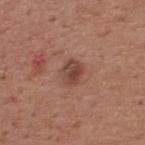notes: no biopsy performed (imaged during a skin exam) | lighting: white-light illumination | imaging modality: ~15 mm crop, total-body skin-cancer survey | patient: male, aged approximately 65 | site: the upper back | lesion size: ~3 mm (longest diameter).